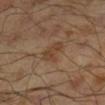Clinical impression: Captured during whole-body skin photography for melanoma surveillance; the lesion was not biopsied. Context: From the right lower leg. A male patient, in their mid-40s. Automated tile analysis of the lesion measured a lesion area of about 5 mm², an eccentricity of roughly 0.75, and a shape-asymmetry score of about 0.25 (0 = symmetric). And it measured a lesion color around L≈40 a*≈17 b*≈29 in CIELAB, about 7 CIELAB-L* units darker than the surrounding skin, and a normalized lesion–skin contrast near 6. The analysis additionally found a classifier nevus-likeness of about 5/100 and lesion-presence confidence of about 100/100. Imaged with cross-polarized lighting. A close-up tile cropped from a whole-body skin photograph, about 15 mm across. The recorded lesion diameter is about 3 mm.